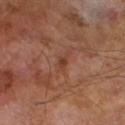Q: Is there a histopathology result?
A: imaged on a skin check; not biopsied
Q: What kind of image is this?
A: ~15 mm tile from a whole-body skin photo
Q: How was the tile lit?
A: cross-polarized
Q: Automated lesion metrics?
A: an area of roughly 2.5 mm², a shape eccentricity near 0.85, and a symmetry-axis asymmetry near 0.4; an average lesion color of about L≈39 a*≈23 b*≈29 (CIELAB), about 7 CIELAB-L* units darker than the surrounding skin, and a normalized border contrast of about 6
Q: Lesion size?
A: ~2.5 mm (longest diameter)
Q: Patient demographics?
A: male, in their mid- to late 60s